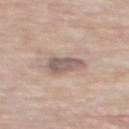The lesion was tiled from a total-body skin photograph and was not biopsied.
Located on the mid back.
Cropped from a total-body skin-imaging series; the visible field is about 15 mm.
The tile uses white-light illumination.
The lesion's longest dimension is about 4 mm.
Automated tile analysis of the lesion measured an outline eccentricity of about 0.8 (0 = round, 1 = elongated) and a symmetry-axis asymmetry near 0.35.
A male subject in their 70s.